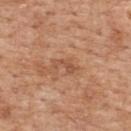Impression: Recorded during total-body skin imaging; not selected for excision or biopsy.